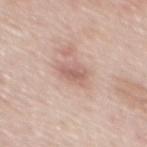Assessment:
The lesion was photographed on a routine skin check and not biopsied; there is no pathology result.
Image and clinical context:
A male subject aged approximately 55. The lesion-visualizer software estimated a normalized lesion–skin contrast near 6. It also reported an automated nevus-likeness rating near 10 out of 100 and a lesion-detection confidence of about 100/100. From the mid back. This image is a 15 mm lesion crop taken from a total-body photograph. Captured under white-light illumination.